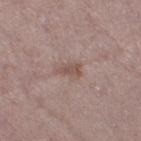Impression:
This lesion was catalogued during total-body skin photography and was not selected for biopsy.
Context:
Located on the right thigh. A lesion tile, about 15 mm wide, cut from a 3D total-body photograph. Automated tile analysis of the lesion measured peripheral color asymmetry of about 0.5. Captured under white-light illumination. The recorded lesion diameter is about 3 mm. A female patient, approximately 40 years of age.